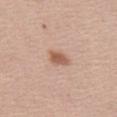The lesion was photographed on a routine skin check and not biopsied; there is no pathology result. On the left thigh. This image is a 15 mm lesion crop taken from a total-body photograph. A female patient aged around 40. About 2.5 mm across.